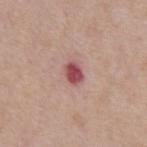| field | value |
|---|---|
| notes | catalogued during a skin exam; not biopsied |
| lesion size | ≈3 mm |
| patient | male, aged 78 to 82 |
| acquisition | ~15 mm crop, total-body skin-cancer survey |
| TBP lesion metrics | roughly 15 lightness units darker than nearby skin and a normalized border contrast of about 10 |
| site | the chest |
| lighting | white-light illumination |